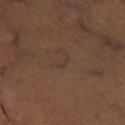Clinical impression:
Imaged during a routine full-body skin examination; the lesion was not biopsied and no histopathology is available.
Clinical summary:
The patient is a male roughly 50 years of age. The lesion is on the left lower leg. A region of skin cropped from a whole-body photographic capture, roughly 15 mm wide.